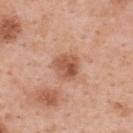{
  "biopsy_status": "not biopsied; imaged during a skin examination",
  "site": "upper back",
  "image": {
    "source": "total-body photography crop",
    "field_of_view_mm": 15
  },
  "patient": {
    "sex": "female",
    "age_approx": 50
  }
}